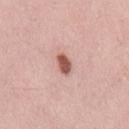Case summary:
* notes — total-body-photography surveillance lesion; no biopsy
* body site — the back
* tile lighting — white-light
* patient — male, aged 33 to 37
* image — total-body-photography crop, ~15 mm field of view